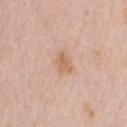lesion_size:
  long_diameter_mm_approx: 2.5
site: chest
lighting: white-light
image:
  source: total-body photography crop
  field_of_view_mm: 15
patient:
  sex: male
  age_approx: 60